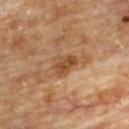notes: no biopsy performed (imaged during a skin exam)
subject: male, aged 83 or over
automated metrics: a shape eccentricity near 0.6 and a symmetry-axis asymmetry near 0.35
site: the upper back
lesion size: ~3 mm (longest diameter)
imaging modality: ~15 mm tile from a whole-body skin photo